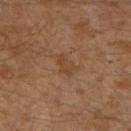Clinical impression: No biopsy was performed on this lesion — it was imaged during a full skin examination and was not determined to be concerning. Acquisition and patient details: On the left leg. Captured under cross-polarized illumination. A close-up tile cropped from a whole-body skin photograph, about 15 mm across. Longest diameter approximately 2.5 mm. A male patient, aged 28–32.